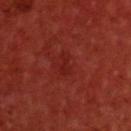biopsy_status: not biopsied; imaged during a skin examination
patient:
  sex: male
  age_approx: 60
site: upper back
image:
  source: total-body photography crop
  field_of_view_mm: 15
lighting: cross-polarized
automated_metrics:
  area_mm2_approx: 3.5
  eccentricity: 0.85
  shape_asymmetry: 0.35
  cielab_L: 24
  cielab_a: 31
  cielab_b: 28
  vs_skin_contrast_norm: 4.5
  border_irregularity_0_10: 4.5
  peripheral_color_asymmetry: 0.5
  nevus_likeness_0_100: 0
  lesion_detection_confidence_0_100: 95
lesion_size:
  long_diameter_mm_approx: 3.0